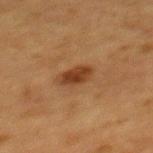On the upper back.
A female patient in their mid- to late 50s.
A close-up tile cropped from a whole-body skin photograph, about 15 mm across.
Captured under cross-polarized illumination.
Longest diameter approximately 3.5 mm.